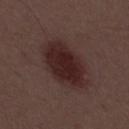workup: imaged on a skin check; not biopsied | lesion size: ≈7 mm | body site: the mid back | subject: male, aged 53–57 | tile lighting: white-light illumination | image: total-body-photography crop, ~15 mm field of view.